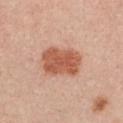The lesion is located on the chest. A female subject, roughly 40 years of age. This image is a 15 mm lesion crop taken from a total-body photograph.